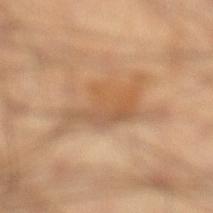biopsy_status: not biopsied; imaged during a skin examination
site: left lower leg
lighting: cross-polarized
automated_metrics:
  area_mm2_approx: 13.0
  eccentricity: 0.9
  border_irregularity_0_10: 7.5
  peripheral_color_asymmetry: 2.0
  nevus_likeness_0_100: 0
  lesion_detection_confidence_0_100: 85
lesion_size:
  long_diameter_mm_approx: 7.0
image:
  source: total-body photography crop
  field_of_view_mm: 15
patient:
  sex: male
  age_approx: 40